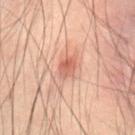The lesion was tiled from a total-body skin photograph and was not biopsied.